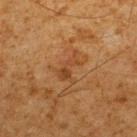Captured during whole-body skin photography for melanoma surveillance; the lesion was not biopsied. Measured at roughly 4 mm in maximum diameter. A 15 mm close-up tile from a total-body photography series done for melanoma screening. Imaged with cross-polarized lighting. The lesion is located on the upper back. A male patient in their 60s.